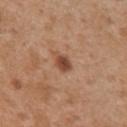Clinical impression:
Recorded during total-body skin imaging; not selected for excision or biopsy.
Acquisition and patient details:
The lesion is on the right upper arm. A lesion tile, about 15 mm wide, cut from a 3D total-body photograph. Measured at roughly 2.5 mm in maximum diameter. Imaged with white-light lighting. A female patient approximately 30 years of age.